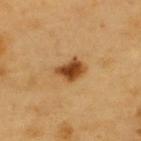Findings:
* follow-up · no biopsy performed (imaged during a skin exam)
* subject · male, aged approximately 60
* image source · ~15 mm tile from a whole-body skin photo
* anatomic site · the back